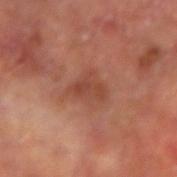image source: total-body-photography crop, ~15 mm field of view
illumination: cross-polarized illumination
lesion diameter: about 3.5 mm
patient: male, aged 63–67
location: the left forearm